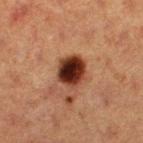| feature | finding |
|---|---|
| follow-up | no biopsy performed (imaged during a skin exam) |
| image source | 15 mm crop, total-body photography |
| illumination | cross-polarized illumination |
| patient | female, roughly 40 years of age |
| TBP lesion metrics | a footprint of about 10 mm², a shape eccentricity near 0.5, and two-axis asymmetry of about 0.15; a lesion color around L≈28 a*≈22 b*≈26 in CIELAB; a nevus-likeness score of about 100/100 and a detector confidence of about 100 out of 100 that the crop contains a lesion |
| lesion size | ~4 mm (longest diameter) |
| location | the left thigh |